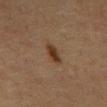Assessment:
The lesion was tiled from a total-body skin photograph and was not biopsied.
Clinical summary:
The lesion is on the mid back. An algorithmic analysis of the crop reported an area of roughly 4.5 mm². And it measured a border-irregularity rating of about 2.5/10. A 15 mm close-up tile from a total-body photography series done for melanoma screening. A male patient, aged 58–62.